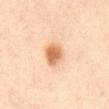Part of a total-body skin-imaging series; this lesion was reviewed on a skin check and was not flagged for biopsy. The subject is a male aged 63 to 67. The lesion is located on the abdomen. Imaged with cross-polarized lighting. A 15 mm crop from a total-body photograph taken for skin-cancer surveillance.